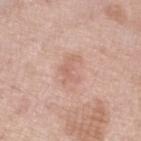Q: Was a biopsy performed?
A: no biopsy performed (imaged during a skin exam)
Q: Lesion location?
A: the left lower leg
Q: What is the lesion's diameter?
A: ≈3 mm
Q: What kind of image is this?
A: 15 mm crop, total-body photography
Q: Patient demographics?
A: female, about 55 years old